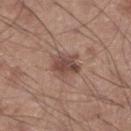Q: Was this lesion biopsied?
A: imaged on a skin check; not biopsied
Q: Lesion location?
A: the right lower leg
Q: Lesion size?
A: ≈3.5 mm
Q: Illumination type?
A: white-light
Q: Automated lesion metrics?
A: a lesion area of about 7 mm², an outline eccentricity of about 0.75 (0 = round, 1 = elongated), and a symmetry-axis asymmetry near 0.3; an average lesion color of about L≈46 a*≈19 b*≈23 (CIELAB) and roughly 11 lightness units darker than nearby skin; a classifier nevus-likeness of about 70/100 and a lesion-detection confidence of about 100/100
Q: What are the patient's age and sex?
A: male, aged around 60
Q: How was this image acquired?
A: total-body-photography crop, ~15 mm field of view